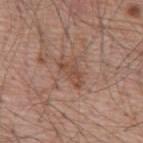– notes · total-body-photography surveillance lesion; no biopsy
– tile lighting · white-light
– image · 15 mm crop, total-body photography
– automated metrics · an area of roughly 6 mm², an outline eccentricity of about 0.85 (0 = round, 1 = elongated), and two-axis asymmetry of about 0.45; a lesion color around L≈49 a*≈21 b*≈27 in CIELAB, about 8 CIELAB-L* units darker than the surrounding skin, and a normalized border contrast of about 6; a color-variation rating of about 2/10 and a peripheral color-asymmetry measure near 1; lesion-presence confidence of about 100/100
– location · the mid back
– lesion diameter · about 4 mm
– subject · male, approximately 55 years of age